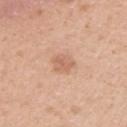Recorded during total-body skin imaging; not selected for excision or biopsy. The recorded lesion diameter is about 2.5 mm. This is a white-light tile. A close-up tile cropped from a whole-body skin photograph, about 15 mm across. An algorithmic analysis of the crop reported a lesion area of about 4.5 mm², a shape eccentricity near 0.3, and two-axis asymmetry of about 0.2. The software also gave a lesion color around L≈63 a*≈22 b*≈32 in CIELAB and roughly 8 lightness units darker than nearby skin. It also reported a border-irregularity index near 2/10 and a within-lesion color-variation index near 3/10. The analysis additionally found a nevus-likeness score of about 20/100 and a lesion-detection confidence of about 100/100. A female subject, aged 43 to 47. The lesion is on the left upper arm.